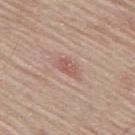This lesion was catalogued during total-body skin photography and was not selected for biopsy.
Longest diameter approximately 2.5 mm.
The patient is a male in their 80s.
Captured under white-light illumination.
A close-up tile cropped from a whole-body skin photograph, about 15 mm across.
Located on the mid back.